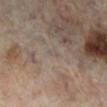– notes — catalogued during a skin exam; not biopsied
– subject — female, aged 58–62
– location — the leg
– image — 15 mm crop, total-body photography